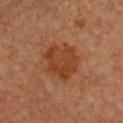Clinical impression: The lesion was tiled from a total-body skin photograph and was not biopsied. Acquisition and patient details: A female patient, roughly 50 years of age. Automated tile analysis of the lesion measured a symmetry-axis asymmetry near 0.2. It also reported a lesion color around L≈34 a*≈22 b*≈31 in CIELAB, a lesion–skin lightness drop of about 7, and a normalized lesion–skin contrast near 7.5. It also reported a nevus-likeness score of about 45/100 and lesion-presence confidence of about 100/100. Located on the chest. A region of skin cropped from a whole-body photographic capture, roughly 15 mm wide.